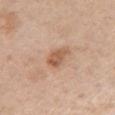Impression:
No biopsy was performed on this lesion — it was imaged during a full skin examination and was not determined to be concerning.